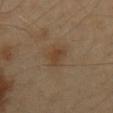biopsy status = no biopsy performed (imaged during a skin exam)
image = 15 mm crop, total-body photography
site = the mid back
patient = male, aged 38–42